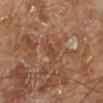The lesion was tiled from a total-body skin photograph and was not biopsied. The lesion-visualizer software estimated an average lesion color of about L≈42 a*≈22 b*≈32 (CIELAB), roughly 6 lightness units darker than nearby skin, and a normalized lesion–skin contrast near 5.5. And it measured a within-lesion color-variation index near 0/10 and a peripheral color-asymmetry measure near 0. And it measured a lesion-detection confidence of about 100/100. The lesion is located on the left lower leg. A male patient aged approximately 70. Measured at roughly 2.5 mm in maximum diameter. Captured under cross-polarized illumination. A 15 mm close-up extracted from a 3D total-body photography capture.